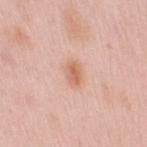<lesion>
<biopsy_status>not biopsied; imaged during a skin examination</biopsy_status>
<patient>
  <sex>female</sex>
  <age_approx>40</age_approx>
</patient>
<lighting>white-light</lighting>
<image>
  <source>total-body photography crop</source>
  <field_of_view_mm>15</field_of_view_mm>
</image>
<site>right upper arm</site>
</lesion>